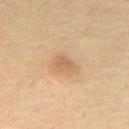Q: Was this lesion biopsied?
A: catalogued during a skin exam; not biopsied
Q: Illumination type?
A: cross-polarized
Q: Patient demographics?
A: male, approximately 70 years of age
Q: Where on the body is the lesion?
A: the back
Q: What kind of image is this?
A: ~15 mm crop, total-body skin-cancer survey